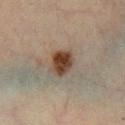Recorded during total-body skin imaging; not selected for excision or biopsy.
A roughly 15 mm field-of-view crop from a total-body skin photograph.
Located on the left lower leg.
The total-body-photography lesion software estimated a lesion area of about 7.5 mm², an eccentricity of roughly 0.55, and a symmetry-axis asymmetry near 0.15. The software also gave a mean CIELAB color near L≈35 a*≈15 b*≈24 and a lesion–skin lightness drop of about 13. It also reported border irregularity of about 1.5 on a 0–10 scale and internal color variation of about 5 on a 0–10 scale.
A male patient roughly 45 years of age.